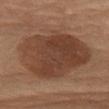Impression:
The lesion was tiled from a total-body skin photograph and was not biopsied.
Image and clinical context:
The lesion is on the left thigh. Imaged with cross-polarized lighting. The patient is a female approximately 70 years of age. A 15 mm close-up tile from a total-body photography series done for melanoma screening. Measured at roughly 8.5 mm in maximum diameter.